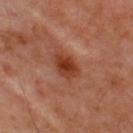From the upper back.
A 15 mm close-up extracted from a 3D total-body photography capture.
A male subject, aged 48–52.
Measured at roughly 3.5 mm in maximum diameter.
The tile uses cross-polarized illumination.
The lesion-visualizer software estimated an area of roughly 6.5 mm² and two-axis asymmetry of about 0.3. It also reported a classifier nevus-likeness of about 80/100 and a detector confidence of about 100 out of 100 that the crop contains a lesion.